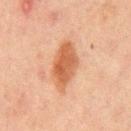Clinical impression: No biopsy was performed on this lesion — it was imaged during a full skin examination and was not determined to be concerning. Clinical summary: On the mid back. Cropped from a total-body skin-imaging series; the visible field is about 15 mm. The subject is a male aged 63 to 67.